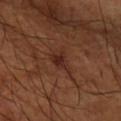A close-up tile cropped from a whole-body skin photograph, about 15 mm across. A male patient aged 63 to 67. This is a cross-polarized tile. The total-body-photography lesion software estimated a lesion area of about 5 mm², a shape eccentricity near 0.6, and a shape-asymmetry score of about 0.4 (0 = symmetric). The software also gave an automated nevus-likeness rating near 10 out of 100. From the right forearm.